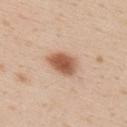{"biopsy_status": "not biopsied; imaged during a skin examination", "image": {"source": "total-body photography crop", "field_of_view_mm": 15}, "lighting": "white-light", "site": "upper back", "lesion_size": {"long_diameter_mm_approx": 4.0}, "patient": {"sex": "male", "age_approx": 25}, "automated_metrics": {"area_mm2_approx": 8.0, "eccentricity": 0.75, "shape_asymmetry": 0.2, "cielab_L": 57, "cielab_a": 22, "cielab_b": 32, "border_irregularity_0_10": 1.5, "peripheral_color_asymmetry": 1.0}}